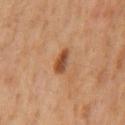Q: How large is the lesion?
A: ≈3 mm
Q: Lesion location?
A: the back
Q: Automated lesion metrics?
A: a lesion area of about 3.5 mm², an eccentricity of roughly 0.85, and a symmetry-axis asymmetry near 0.25; a border-irregularity index near 2.5/10, a color-variation rating of about 4/10, and peripheral color asymmetry of about 1.5
Q: What is the imaging modality?
A: ~15 mm crop, total-body skin-cancer survey
Q: What are the patient's age and sex?
A: male, about 60 years old
Q: Illumination type?
A: cross-polarized